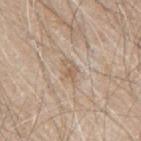Imaged during a routine full-body skin examination; the lesion was not biopsied and no histopathology is available. Measured at roughly 3 mm in maximum diameter. From the mid back. A male patient, about 80 years old. A 15 mm close-up tile from a total-body photography series done for melanoma screening.